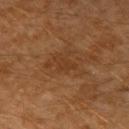Assessment: The lesion was photographed on a routine skin check and not biopsied; there is no pathology result. Background: The lesion is located on the right upper arm. A 15 mm close-up extracted from a 3D total-body photography capture. A male patient approximately 60 years of age.